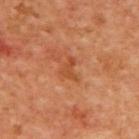notes: imaged on a skin check; not biopsied | TBP lesion metrics: border irregularity of about 6.5 on a 0–10 scale, internal color variation of about 0 on a 0–10 scale, and radial color variation of about 0; a nevus-likeness score of about 0/100 and a detector confidence of about 100 out of 100 that the crop contains a lesion | size: ≈2.5 mm | subject: female, roughly 40 years of age | site: the upper back | tile lighting: cross-polarized | image: ~15 mm tile from a whole-body skin photo.